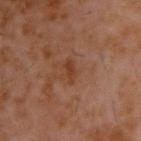The lesion was tiled from a total-body skin photograph and was not biopsied. A male subject, aged 58–62. From the upper back. A close-up tile cropped from a whole-body skin photograph, about 15 mm across.